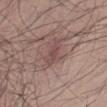Clinical impression: The lesion was tiled from a total-body skin photograph and was not biopsied. Clinical summary: Approximately 3.5 mm at its widest. A male subject, roughly 30 years of age. Cropped from a whole-body photographic skin survey; the tile spans about 15 mm. Imaged with white-light lighting. An algorithmic analysis of the crop reported a footprint of about 5 mm², a shape eccentricity near 0.85, and a symmetry-axis asymmetry near 0.35. It also reported a border-irregularity rating of about 4.5/10 and a peripheral color-asymmetry measure near 0.5. The analysis additionally found a nevus-likeness score of about 0/100 and lesion-presence confidence of about 95/100. Located on the left lower leg.